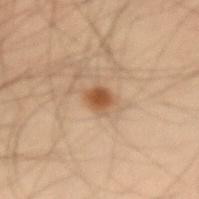Captured during whole-body skin photography for melanoma surveillance; the lesion was not biopsied.
On the right forearm.
The total-body-photography lesion software estimated an area of roughly 4.5 mm², a shape eccentricity near 0.55, and two-axis asymmetry of about 0.25. The software also gave a nevus-likeness score of about 95/100 and a lesion-detection confidence of about 100/100.
Captured under cross-polarized illumination.
The subject is a male aged around 55.
A roughly 15 mm field-of-view crop from a total-body skin photograph.